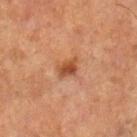workup: catalogued during a skin exam; not biopsied | lesion size: ~2.5 mm (longest diameter) | location: the leg | subject: male, about 65 years old | acquisition: ~15 mm crop, total-body skin-cancer survey | TBP lesion metrics: a lesion color around L≈39 a*≈21 b*≈30 in CIELAB, roughly 9 lightness units darker than nearby skin, and a normalized border contrast of about 8.